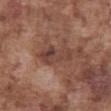Q: What are the patient's age and sex?
A: male, aged around 75
Q: What is the imaging modality?
A: ~15 mm tile from a whole-body skin photo
Q: How was the tile lit?
A: white-light
Q: What is the anatomic site?
A: the front of the torso